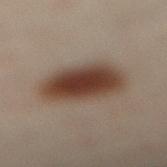Q: Was a biopsy performed?
A: total-body-photography surveillance lesion; no biopsy
Q: Where on the body is the lesion?
A: the left leg
Q: What did automated image analysis measure?
A: a footprint of about 21 mm², a shape eccentricity near 0.45, and a shape-asymmetry score of about 0.15 (0 = symmetric); a border-irregularity rating of about 1.5/10, a within-lesion color-variation index near 4.5/10, and radial color variation of about 1; a detector confidence of about 100 out of 100 that the crop contains a lesion
Q: Illumination type?
A: cross-polarized
Q: Who is the patient?
A: male, aged 48 to 52
Q: How large is the lesion?
A: ≈5.5 mm
Q: What kind of image is this?
A: ~15 mm tile from a whole-body skin photo